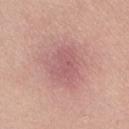Captured during whole-body skin photography for melanoma surveillance; the lesion was not biopsied. Automated tile analysis of the lesion measured a lesion area of about 9.5 mm², an eccentricity of roughly 0.65, and two-axis asymmetry of about 0.25. It also reported a mean CIELAB color near L≈57 a*≈25 b*≈21, about 7 CIELAB-L* units darker than the surrounding skin, and a normalized border contrast of about 5. A female patient about 45 years old. The lesion is located on the lower back. Approximately 4 mm at its widest. Captured under white-light illumination. A 15 mm close-up tile from a total-body photography series done for melanoma screening.